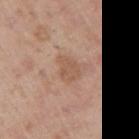biopsy status: imaged on a skin check; not biopsied | TBP lesion metrics: a mean CIELAB color near L≈55 a*≈19 b*≈30, roughly 7 lightness units darker than nearby skin, and a normalized lesion–skin contrast near 5.5 | location: the right upper arm | image source: ~15 mm tile from a whole-body skin photo | subject: male, approximately 40 years of age.